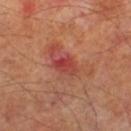Assessment:
No biopsy was performed on this lesion — it was imaged during a full skin examination and was not determined to be concerning.
Background:
A male patient aged around 70. Automated tile analysis of the lesion measured an area of roughly 5.5 mm², an outline eccentricity of about 0.7 (0 = round, 1 = elongated), and two-axis asymmetry of about 0.25. The software also gave an average lesion color of about L≈43 a*≈33 b*≈28 (CIELAB), a lesion–skin lightness drop of about 9, and a normalized border contrast of about 7. The analysis additionally found a border-irregularity index near 2.5/10, internal color variation of about 6 on a 0–10 scale, and peripheral color asymmetry of about 2. And it measured an automated nevus-likeness rating near 0 out of 100 and a detector confidence of about 100 out of 100 that the crop contains a lesion. Cropped from a total-body skin-imaging series; the visible field is about 15 mm. Located on the right lower leg. This is a cross-polarized tile. Approximately 3 mm at its widest.